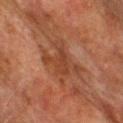Part of a total-body skin-imaging series; this lesion was reviewed on a skin check and was not flagged for biopsy. Imaged with cross-polarized lighting. The lesion is on the upper back. About 6 mm across. Cropped from a total-body skin-imaging series; the visible field is about 15 mm. A male subject, aged 73 to 77.